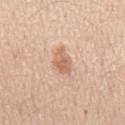Q: Is there a histopathology result?
A: total-body-photography surveillance lesion; no biopsy
Q: Illumination type?
A: white-light illumination
Q: What is the imaging modality?
A: 15 mm crop, total-body photography
Q: What are the patient's age and sex?
A: male, in their 60s
Q: Lesion location?
A: the chest
Q: What did automated image analysis measure?
A: a mean CIELAB color near L≈64 a*≈20 b*≈31 and about 11 CIELAB-L* units darker than the surrounding skin; a classifier nevus-likeness of about 50/100 and a detector confidence of about 100 out of 100 that the crop contains a lesion
Q: How large is the lesion?
A: ≈4 mm